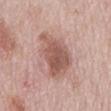{
  "image": {
    "source": "total-body photography crop",
    "field_of_view_mm": 15
  },
  "site": "mid back",
  "lesion_size": {
    "long_diameter_mm_approx": 6.5
  },
  "lighting": "white-light",
  "patient": {
    "sex": "male",
    "age_approx": 75
  }
}